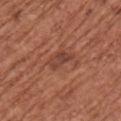biopsy status: no biopsy performed (imaged during a skin exam).